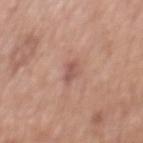notes: no biopsy performed (imaged during a skin exam)
patient: male, aged approximately 70
location: the mid back
diameter: about 2.5 mm
image: ~15 mm tile from a whole-body skin photo
lighting: white-light illumination
automated metrics: a classifier nevus-likeness of about 0/100 and lesion-presence confidence of about 100/100